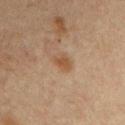Case summary:
- workup: total-body-photography surveillance lesion; no biopsy
- subject: male, approximately 60 years of age
- diameter: about 2.5 mm
- image source: ~15 mm tile from a whole-body skin photo
- lighting: cross-polarized
- image-analysis metrics: an area of roughly 4 mm², a shape eccentricity near 0.7, and two-axis asymmetry of about 0.25; an average lesion color of about L≈42 a*≈16 b*≈29 (CIELAB), roughly 7 lightness units darker than nearby skin, and a lesion-to-skin contrast of about 7 (normalized; higher = more distinct)
- body site: the left upper arm